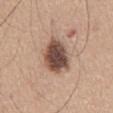workup: no biopsy performed (imaged during a skin exam)
body site: the abdomen
tile lighting: white-light illumination
image source: ~15 mm crop, total-body skin-cancer survey
lesion diameter: about 5.5 mm
automated metrics: a footprint of about 13 mm², a shape eccentricity near 0.8, and a shape-asymmetry score of about 0.15 (0 = symmetric); a normalized border contrast of about 13; a within-lesion color-variation index near 5/10
patient: male, aged around 65